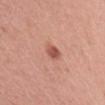The tile uses white-light illumination.
A male patient about 60 years old.
On the right upper arm.
An algorithmic analysis of the crop reported a footprint of about 5 mm², an eccentricity of roughly 0.35, and two-axis asymmetry of about 0.25. The software also gave a mean CIELAB color near L≈56 a*≈26 b*≈29, a lesion–skin lightness drop of about 10, and a lesion-to-skin contrast of about 7 (normalized; higher = more distinct).
Approximately 2.5 mm at its widest.
Cropped from a total-body skin-imaging series; the visible field is about 15 mm.